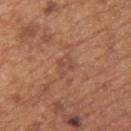The lesion was photographed on a routine skin check and not biopsied; there is no pathology result.
A region of skin cropped from a whole-body photographic capture, roughly 15 mm wide.
Longest diameter approximately 2.5 mm.
Automated image analysis of the tile measured a border-irregularity rating of about 3/10, internal color variation of about 1.5 on a 0–10 scale, and a peripheral color-asymmetry measure near 0.5.
A male subject approximately 65 years of age.
Located on the upper back.